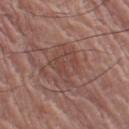Imaged during a routine full-body skin examination; the lesion was not biopsied and no histopathology is available. Captured under white-light illumination. A female patient aged 78–82. Cropped from a whole-body photographic skin survey; the tile spans about 15 mm. From the left thigh.